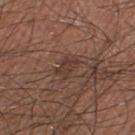Clinical impression:
The lesion was photographed on a routine skin check and not biopsied; there is no pathology result.
Image and clinical context:
Automated image analysis of the tile measured an area of roughly 4.5 mm², a shape eccentricity near 0.8, and a symmetry-axis asymmetry near 0.3. The analysis additionally found about 7 CIELAB-L* units darker than the surrounding skin and a normalized border contrast of about 6. The software also gave border irregularity of about 3.5 on a 0–10 scale and a within-lesion color-variation index near 1.5/10. A roughly 15 mm field-of-view crop from a total-body skin photograph. Imaged with white-light lighting. The lesion's longest dimension is about 3 mm. A male patient aged around 60. On the right lower leg.